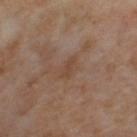Impression: Imaged during a routine full-body skin examination; the lesion was not biopsied and no histopathology is available. Image and clinical context: Measured at roughly 2.5 mm in maximum diameter. The subject is a female aged around 55. The lesion-visualizer software estimated a normalized border contrast of about 5. And it measured a border-irregularity index near 3.5/10, a color-variation rating of about 0/10, and radial color variation of about 0. From the left thigh. Imaged with cross-polarized lighting. Cropped from a whole-body photographic skin survey; the tile spans about 15 mm.